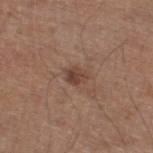<record>
<biopsy_status>not biopsied; imaged during a skin examination</biopsy_status>
<lighting>white-light</lighting>
<image>
  <source>total-body photography crop</source>
  <field_of_view_mm>15</field_of_view_mm>
</image>
<automated_metrics>
  <cielab_L>42</cielab_L>
  <cielab_a>19</cielab_a>
  <cielab_b>25</cielab_b>
  <vs_skin_darker_L>9.0</vs_skin_darker_L>
  <vs_skin_contrast_norm>7.5</vs_skin_contrast_norm>
  <color_variation_0_10>2.0</color_variation_0_10>
  <peripheral_color_asymmetry>1.0</peripheral_color_asymmetry>
  <nevus_likeness_0_100>30</nevus_likeness_0_100>
  <lesion_detection_confidence_0_100>100</lesion_detection_confidence_0_100>
</automated_metrics>
<patient>
  <sex>male</sex>
  <age_approx>70</age_approx>
</patient>
<site>right thigh</site>
</record>